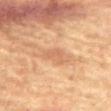This lesion was catalogued during total-body skin photography and was not selected for biopsy.
Captured under cross-polarized illumination.
The subject is a male about 65 years old.
Located on the abdomen.
The lesion's longest dimension is about 3 mm.
This image is a 15 mm lesion crop taken from a total-body photograph.